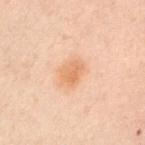No biopsy was performed on this lesion — it was imaged during a full skin examination and was not determined to be concerning.
A 15 mm crop from a total-body photograph taken for skin-cancer surveillance.
Captured under cross-polarized illumination.
From the left upper arm.
Automated image analysis of the tile measured a lesion–skin lightness drop of about 7. It also reported a color-variation rating of about 2.5/10 and radial color variation of about 1.
A male subject, aged around 50.